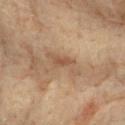– follow-up · imaged on a skin check; not biopsied
– body site · the upper back
– diameter · ≈4.5 mm
– automated metrics · a footprint of about 5.5 mm² and a shape-asymmetry score of about 0.5 (0 = symmetric); a classifier nevus-likeness of about 0/100 and a lesion-detection confidence of about 60/100
– acquisition · total-body-photography crop, ~15 mm field of view
– tile lighting · cross-polarized
– patient · female, roughly 80 years of age